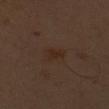size=about 2.5 mm | anatomic site=the left upper arm | tile lighting=cross-polarized | patient=male, approximately 50 years of age | acquisition=~15 mm tile from a whole-body skin photo.